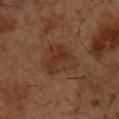Assessment: Captured during whole-body skin photography for melanoma surveillance; the lesion was not biopsied. Background: This image is a 15 mm lesion crop taken from a total-body photograph. The patient is a male approximately 55 years of age. The lesion is located on the chest.